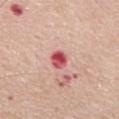size: about 2.5 mm
image-analysis metrics: a lesion color around L≈54 a*≈38 b*≈24 in CIELAB and roughly 17 lightness units darker than nearby skin; a peripheral color-asymmetry measure near 3; a classifier nevus-likeness of about 0/100 and a lesion-detection confidence of about 100/100
anatomic site: the back
patient: male, in their mid-70s
tile lighting: white-light
acquisition: ~15 mm crop, total-body skin-cancer survey